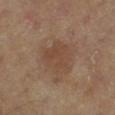Q: Is there a histopathology result?
A: catalogued during a skin exam; not biopsied
Q: What kind of image is this?
A: ~15 mm crop, total-body skin-cancer survey
Q: How was the tile lit?
A: cross-polarized illumination
Q: Who is the patient?
A: male, aged around 65
Q: How large is the lesion?
A: ~5 mm (longest diameter)
Q: Lesion location?
A: the leg